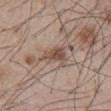Assessment: Captured during whole-body skin photography for melanoma surveillance; the lesion was not biopsied. Image and clinical context: Longest diameter approximately 4 mm. A male patient, in their mid-50s. Imaged with white-light lighting. A close-up tile cropped from a whole-body skin photograph, about 15 mm across. Located on the front of the torso. Automated image analysis of the tile measured a mean CIELAB color near L≈50 a*≈16 b*≈24, roughly 10 lightness units darker than nearby skin, and a normalized border contrast of about 7.5.